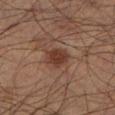Clinical impression:
Recorded during total-body skin imaging; not selected for excision or biopsy.
Acquisition and patient details:
The lesion is on the left lower leg. Measured at roughly 3.5 mm in maximum diameter. A 15 mm close-up extracted from a 3D total-body photography capture. A male subject in their 50s.